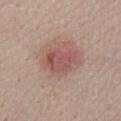Findings:
• lesion diameter: ~4 mm (longest diameter)
• patient: male, approximately 40 years of age
• body site: the back
• image source: total-body-photography crop, ~15 mm field of view
• image-analysis metrics: a lesion area of about 10 mm² and a shape eccentricity near 0.65; a border-irregularity rating of about 2.5/10, a color-variation rating of about 4/10, and peripheral color asymmetry of about 1
• lighting: white-light